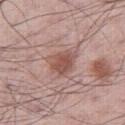{"biopsy_status": "not biopsied; imaged during a skin examination", "patient": {"sex": "male", "age_approx": 60}, "lesion_size": {"long_diameter_mm_approx": 4.0}, "image": {"source": "total-body photography crop", "field_of_view_mm": 15}, "automated_metrics": {"area_mm2_approx": 9.0, "eccentricity": 0.55, "shape_asymmetry": 0.35, "cielab_L": 53, "cielab_a": 21, "cielab_b": 24, "vs_skin_darker_L": 10.0, "color_variation_0_10": 3.5, "peripheral_color_asymmetry": 1.0, "nevus_likeness_0_100": 80, "lesion_detection_confidence_0_100": 100}, "site": "right thigh", "lighting": "white-light"}